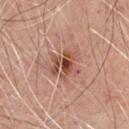Case summary:
* biopsy status — catalogued during a skin exam; not biopsied
* patient — male, aged 63 to 67
* anatomic site — the chest
* lesion size — ~4 mm (longest diameter)
* illumination — cross-polarized illumination
* image — 15 mm crop, total-body photography